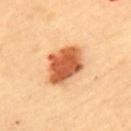Impression: No biopsy was performed on this lesion — it was imaged during a full skin examination and was not determined to be concerning. Image and clinical context: The lesion is located on the upper back. Imaged with cross-polarized lighting. Automated image analysis of the tile measured a border-irregularity index near 2/10 and radial color variation of about 1.5. It also reported an automated nevus-likeness rating near 100 out of 100 and a detector confidence of about 100 out of 100 that the crop contains a lesion. The recorded lesion diameter is about 5.5 mm. A female subject aged around 60. A 15 mm close-up tile from a total-body photography series done for melanoma screening.